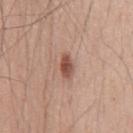{"biopsy_status": "not biopsied; imaged during a skin examination", "patient": {"sex": "male", "age_approx": 55}, "image": {"source": "total-body photography crop", "field_of_view_mm": 15}, "automated_metrics": {"nevus_likeness_0_100": 95, "lesion_detection_confidence_0_100": 100}, "lighting": "white-light", "site": "chest", "lesion_size": {"long_diameter_mm_approx": 3.0}}